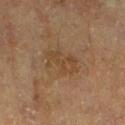Clinical impression: The lesion was tiled from a total-body skin photograph and was not biopsied. Context: Approximately 4.5 mm at its widest. A male subject in their mid- to late 80s or older. A close-up tile cropped from a whole-body skin photograph, about 15 mm across. Imaged with cross-polarized lighting. Located on the leg.